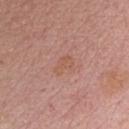The lesion was photographed on a routine skin check and not biopsied; there is no pathology result.